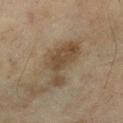Q: Was this lesion biopsied?
A: no biopsy performed (imaged during a skin exam)
Q: How large is the lesion?
A: about 6.5 mm
Q: What did automated image analysis measure?
A: a color-variation rating of about 2.5/10 and a peripheral color-asymmetry measure near 0.5; an automated nevus-likeness rating near 10 out of 100 and a lesion-detection confidence of about 100/100
Q: How was this image acquired?
A: ~15 mm tile from a whole-body skin photo
Q: What is the anatomic site?
A: the left lower leg
Q: What are the patient's age and sex?
A: female, aged around 60
Q: Illumination type?
A: cross-polarized